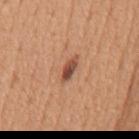biopsy_status: not biopsied; imaged during a skin examination
lighting: white-light
lesion_size:
  long_diameter_mm_approx: 3.5
patient:
  sex: male
  age_approx: 55
image:
  source: total-body photography crop
  field_of_view_mm: 15
site: mid back